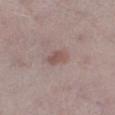Notes:
- workup · total-body-photography surveillance lesion; no biopsy
- location · the left lower leg
- tile lighting · white-light
- diameter · about 3 mm
- acquisition · ~15 mm tile from a whole-body skin photo
- subject · male, approximately 40 years of age